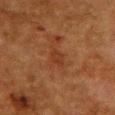Assessment:
Recorded during total-body skin imaging; not selected for excision or biopsy.
Background:
A lesion tile, about 15 mm wide, cut from a 3D total-body photograph. From the chest. A female subject about 50 years old.